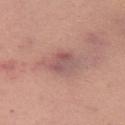<case>
<biopsy_status>not biopsied; imaged during a skin examination</biopsy_status>
<site>right upper arm</site>
<image>
  <source>total-body photography crop</source>
  <field_of_view_mm>15</field_of_view_mm>
</image>
<lighting>white-light</lighting>
<patient>
  <sex>female</sex>
  <age_approx>35</age_approx>
</patient>
</case>